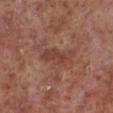Part of a total-body skin-imaging series; this lesion was reviewed on a skin check and was not flagged for biopsy. A close-up tile cropped from a whole-body skin photograph, about 15 mm across. The subject is a male approximately 55 years of age. On the left lower leg. The total-body-photography lesion software estimated a nevus-likeness score of about 0/100 and a lesion-detection confidence of about 100/100.